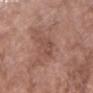<tbp_lesion>
  <biopsy_status>not biopsied; imaged during a skin examination</biopsy_status>
  <image>
    <source>total-body photography crop</source>
    <field_of_view_mm>15</field_of_view_mm>
  </image>
  <lesion_size>
    <long_diameter_mm_approx>4.5</long_diameter_mm_approx>
  </lesion_size>
  <patient>
    <sex>female</sex>
    <age_approx>70</age_approx>
  </patient>
  <automated_metrics>
    <area_mm2_approx>6.5</area_mm2_approx>
    <eccentricity>0.9</eccentricity>
    <shape_asymmetry>0.35</shape_asymmetry>
    <nevus_likeness_0_100>0</nevus_likeness_0_100>
    <lesion_detection_confidence_0_100>85</lesion_detection_confidence_0_100>
  </automated_metrics>
  <site>left forearm</site>
  <lighting>white-light</lighting>
</tbp_lesion>